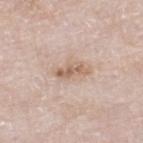<tbp_lesion>
  <biopsy_status>not biopsied; imaged during a skin examination</biopsy_status>
  <site>right lower leg</site>
  <lighting>white-light</lighting>
  <image>
    <source>total-body photography crop</source>
    <field_of_view_mm>15</field_of_view_mm>
  </image>
  <patient>
    <sex>male</sex>
    <age_approx>80</age_approx>
  </patient>
</tbp_lesion>